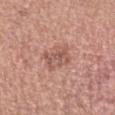Q: What is the imaging modality?
A: ~15 mm tile from a whole-body skin photo
Q: What did automated image analysis measure?
A: a shape eccentricity near 0.45 and two-axis asymmetry of about 0.2; a mean CIELAB color near L≈56 a*≈22 b*≈26, a lesion–skin lightness drop of about 9, and a normalized border contrast of about 6
Q: Patient demographics?
A: female, aged 33–37
Q: What is the anatomic site?
A: the head or neck
Q: How large is the lesion?
A: about 3.5 mm